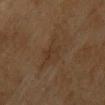Notes:
– workup — imaged on a skin check; not biopsied
– image-analysis metrics — a footprint of about 8 mm²; border irregularity of about 5.5 on a 0–10 scale, a within-lesion color-variation index near 3/10, and a peripheral color-asymmetry measure near 1
– patient — female, aged 53–57
– illumination — cross-polarized
– lesion diameter — ≈4.5 mm
– anatomic site — the front of the torso
– acquisition — 15 mm crop, total-body photography